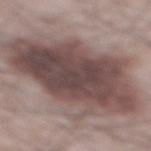follow-up: no biopsy performed (imaged during a skin exam) | acquisition: ~15 mm crop, total-body skin-cancer survey | illumination: white-light | image-analysis metrics: a shape eccentricity near 0.8 | diameter: about 12.5 mm | subject: male, in their mid- to late 60s | location: the mid back.